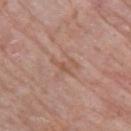Recorded during total-body skin imaging; not selected for excision or biopsy. About 3.5 mm across. The lesion is located on the right upper arm. Captured under white-light illumination. A region of skin cropped from a whole-body photographic capture, roughly 15 mm wide. The subject is a male aged around 85 or older.